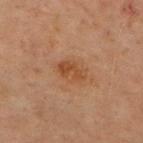Cropped from a whole-body photographic skin survey; the tile spans about 15 mm. A male subject, approximately 70 years of age. Imaged with cross-polarized lighting. The lesion is on the chest. About 3.5 mm across. The lesion-visualizer software estimated a mean CIELAB color near L≈36 a*≈18 b*≈28, a lesion–skin lightness drop of about 6, and a lesion-to-skin contrast of about 6.5 (normalized; higher = more distinct). The software also gave a border-irregularity index near 2/10, a within-lesion color-variation index near 3.5/10, and peripheral color asymmetry of about 1.5.